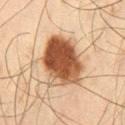Captured during whole-body skin photography for melanoma surveillance; the lesion was not biopsied.
A male patient about 45 years old.
Imaged with cross-polarized lighting.
This image is a 15 mm lesion crop taken from a total-body photograph.
Located on the right thigh.
The lesion-visualizer software estimated a footprint of about 24 mm² and a shape-asymmetry score of about 0.15 (0 = symmetric). It also reported an average lesion color of about L≈43 a*≈19 b*≈30 (CIELAB) and roughly 17 lightness units darker than nearby skin. And it measured a nevus-likeness score of about 100/100.